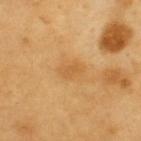biopsy status = total-body-photography surveillance lesion; no biopsy | illumination = cross-polarized illumination | patient = male, about 60 years old | TBP lesion metrics = roughly 7 lightness units darker than nearby skin | imaging modality = ~15 mm tile from a whole-body skin photo | diameter = about 2.5 mm | location = the upper back.